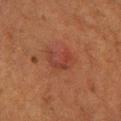biopsy status: catalogued during a skin exam; not biopsied
lighting: cross-polarized illumination
lesion size: ~3 mm (longest diameter)
patient: female, in their 50s
acquisition: 15 mm crop, total-body photography
location: the left lower leg